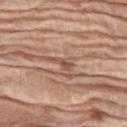Captured during whole-body skin photography for melanoma surveillance; the lesion was not biopsied. A region of skin cropped from a whole-body photographic capture, roughly 15 mm wide. About 3 mm across. A female subject roughly 75 years of age. The total-body-photography lesion software estimated an outline eccentricity of about 0.9 (0 = round, 1 = elongated) and two-axis asymmetry of about 0.55. The software also gave a lesion–skin lightness drop of about 9 and a normalized lesion–skin contrast near 6. The tile uses white-light illumination. Located on the left thigh.